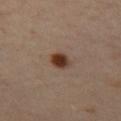<tbp_lesion>
  <biopsy_status>not biopsied; imaged during a skin examination</biopsy_status>
  <patient>
    <sex>female</sex>
    <age_approx>40</age_approx>
  </patient>
  <lesion_size>
    <long_diameter_mm_approx>2.5</long_diameter_mm_approx>
  </lesion_size>
  <site>left thigh</site>
  <lighting>cross-polarized</lighting>
  <image>
    <source>total-body photography crop</source>
    <field_of_view_mm>15</field_of_view_mm>
  </image>
</tbp_lesion>